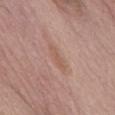Q: What are the patient's age and sex?
A: male, in their 70s
Q: How large is the lesion?
A: about 4 mm
Q: Illumination type?
A: white-light
Q: Lesion location?
A: the abdomen
Q: How was this image acquired?
A: ~15 mm crop, total-body skin-cancer survey